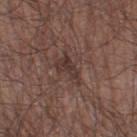Clinical impression:
No biopsy was performed on this lesion — it was imaged during a full skin examination and was not determined to be concerning.
Background:
The lesion is on the right thigh. The patient is a male in their mid- to late 50s. An algorithmic analysis of the crop reported a footprint of about 6 mm². The tile uses white-light illumination. A lesion tile, about 15 mm wide, cut from a 3D total-body photograph. The recorded lesion diameter is about 4.5 mm.